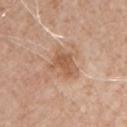• notes — total-body-photography surveillance lesion; no biopsy
• patient — male, in their mid-60s
• image — total-body-photography crop, ~15 mm field of view
• diameter — about 4 mm
• anatomic site — the chest